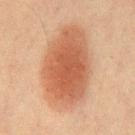Q: Was this lesion biopsied?
A: imaged on a skin check; not biopsied
Q: Lesion location?
A: the chest
Q: What is the imaging modality?
A: 15 mm crop, total-body photography
Q: Who is the patient?
A: male, aged 58 to 62